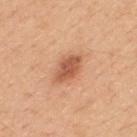The lesion was photographed on a routine skin check and not biopsied; there is no pathology result. Measured at roughly 4 mm in maximum diameter. Captured under white-light illumination. Cropped from a whole-body photographic skin survey; the tile spans about 15 mm. A male subject aged approximately 50. From the upper back.